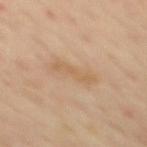<tbp_lesion>
  <biopsy_status>not biopsied; imaged during a skin examination</biopsy_status>
  <lighting>cross-polarized</lighting>
  <image>
    <source>total-body photography crop</source>
    <field_of_view_mm>15</field_of_view_mm>
  </image>
  <site>mid back</site>
  <patient>
    <sex>male</sex>
    <age_approx>55</age_approx>
  </patient>
</tbp_lesion>